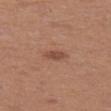biopsy status=total-body-photography surveillance lesion; no biopsy
anatomic site=the left thigh
acquisition=~15 mm crop, total-body skin-cancer survey
subject=female, aged around 40
lesion diameter=about 2.5 mm